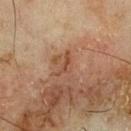* notes — total-body-photography surveillance lesion; no biopsy
* subject — male, roughly 70 years of age
* location — the front of the torso
* imaging modality — total-body-photography crop, ~15 mm field of view
* illumination — cross-polarized illumination